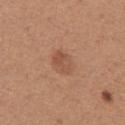workup = catalogued during a skin exam; not biopsied
size = ~3 mm (longest diameter)
TBP lesion metrics = a nevus-likeness score of about 30/100 and a lesion-detection confidence of about 100/100
acquisition = ~15 mm crop, total-body skin-cancer survey
patient = female, aged approximately 40
location = the right upper arm
tile lighting = white-light illumination